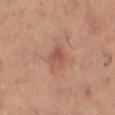notes: no biopsy performed (imaged during a skin exam)
image: ~15 mm tile from a whole-body skin photo
anatomic site: the left thigh
size: about 3 mm
automated metrics: an eccentricity of roughly 0.7 and a shape-asymmetry score of about 0.2 (0 = symmetric); about 8 CIELAB-L* units darker than the surrounding skin and a normalized lesion–skin contrast near 6; a classifier nevus-likeness of about 5/100
patient: female, aged around 40
tile lighting: cross-polarized illumination